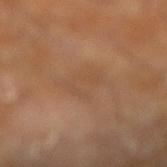Findings:
– workup — no biopsy performed (imaged during a skin exam)
– subject — male, aged 58 to 62
– body site — the leg
– image — 15 mm crop, total-body photography